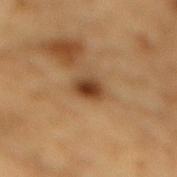follow-up: catalogued during a skin exam; not biopsied | imaging modality: 15 mm crop, total-body photography | image-analysis metrics: a lesion area of about 5 mm², an outline eccentricity of about 0.6 (0 = round, 1 = elongated), and two-axis asymmetry of about 0.2 | body site: the mid back | patient: male, aged around 85 or older | size: about 3 mm | illumination: cross-polarized illumination.